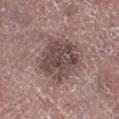{"biopsy_status": "not biopsied; imaged during a skin examination"}